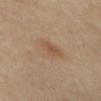Impression: Imaged during a routine full-body skin examination; the lesion was not biopsied and no histopathology is available. Acquisition and patient details: From the abdomen. The patient is a female aged 48–52. A 15 mm crop from a total-body photograph taken for skin-cancer surveillance.